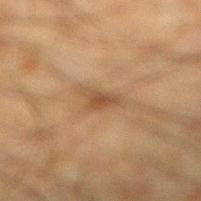Impression:
Captured during whole-body skin photography for melanoma surveillance; the lesion was not biopsied.
Background:
A 15 mm crop from a total-body photograph taken for skin-cancer surveillance. The lesion is located on the right lower leg. The lesion's longest dimension is about 3.5 mm. A male subject in their mid-30s. Imaged with cross-polarized lighting.